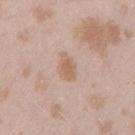Case summary:
- imaging modality: total-body-photography crop, ~15 mm field of view
- site: the left thigh
- diameter: ≈3 mm
- lighting: white-light illumination
- automated metrics: an outline eccentricity of about 0.75 (0 = round, 1 = elongated) and a symmetry-axis asymmetry near 0.3; a border-irregularity rating of about 2.5/10 and peripheral color asymmetry of about 0.5; a classifier nevus-likeness of about 0/100 and a detector confidence of about 100 out of 100 that the crop contains a lesion
- patient: female, aged approximately 25